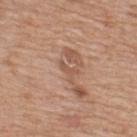Q: Was a biopsy performed?
A: catalogued during a skin exam; not biopsied
Q: How was this image acquired?
A: ~15 mm crop, total-body skin-cancer survey
Q: Where on the body is the lesion?
A: the upper back
Q: What are the patient's age and sex?
A: male, about 60 years old